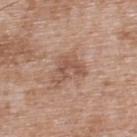Findings:
• notes · imaged on a skin check; not biopsied
• image · total-body-photography crop, ~15 mm field of view
• site · the back
• lighting · white-light
• subject · male, approximately 50 years of age
• size · ~4 mm (longest diameter)